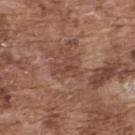Impression:
Recorded during total-body skin imaging; not selected for excision or biopsy.
Background:
Automated tile analysis of the lesion measured a footprint of about 4 mm² and a shape eccentricity near 0.75. The software also gave about 7 CIELAB-L* units darker than the surrounding skin and a lesion-to-skin contrast of about 5.5 (normalized; higher = more distinct). The software also gave a border-irregularity rating of about 5/10, internal color variation of about 1.5 on a 0–10 scale, and peripheral color asymmetry of about 0.5. The analysis additionally found a nevus-likeness score of about 0/100 and a detector confidence of about 90 out of 100 that the crop contains a lesion. A male patient, in their mid- to late 70s. A region of skin cropped from a whole-body photographic capture, roughly 15 mm wide. The lesion is on the back. Measured at roughly 3 mm in maximum diameter. Imaged with white-light lighting.